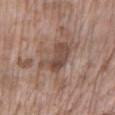Imaged during a routine full-body skin examination; the lesion was not biopsied and no histopathology is available. This image is a 15 mm lesion crop taken from a total-body photograph. On the left lower leg. A female patient approximately 75 years of age. Captured under white-light illumination. Automated tile analysis of the lesion measured about 10 CIELAB-L* units darker than the surrounding skin and a lesion-to-skin contrast of about 7.5 (normalized; higher = more distinct). The software also gave a border-irregularity index near 4.5/10 and radial color variation of about 1. The software also gave a detector confidence of about 100 out of 100 that the crop contains a lesion. Longest diameter approximately 5 mm.